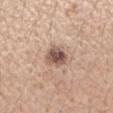* workup: total-body-photography surveillance lesion; no biopsy
* subject: female, about 45 years old
* illumination: white-light illumination
* lesion size: ≈3 mm
* image: ~15 mm tile from a whole-body skin photo
* site: the left forearm